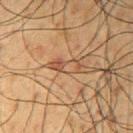Captured during whole-body skin photography for melanoma surveillance; the lesion was not biopsied.
The lesion is located on the right upper arm.
Cropped from a whole-body photographic skin survey; the tile spans about 15 mm.
A male patient, aged 58 to 62.